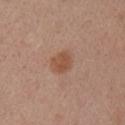Q: Was a biopsy performed?
A: total-body-photography surveillance lesion; no biopsy
Q: What is the anatomic site?
A: the right upper arm
Q: Illumination type?
A: white-light
Q: What is the imaging modality?
A: ~15 mm tile from a whole-body skin photo
Q: How large is the lesion?
A: ~3 mm (longest diameter)
Q: What are the patient's age and sex?
A: female, aged approximately 55
Q: Automated lesion metrics?
A: a lesion area of about 5.5 mm², an outline eccentricity of about 0.5 (0 = round, 1 = elongated), and a shape-asymmetry score of about 0.15 (0 = symmetric); about 9 CIELAB-L* units darker than the surrounding skin and a normalized border contrast of about 7; a color-variation rating of about 2.5/10